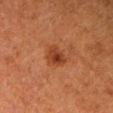follow-up = imaged on a skin check; not biopsied
acquisition = ~15 mm tile from a whole-body skin photo
location = the right upper arm
subject = female, aged 48 to 52
lesion size = ≈3 mm